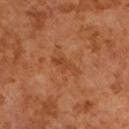notes = imaged on a skin check; not biopsied | illumination = cross-polarized | subject = male, approximately 65 years of age | size = ≈3.5 mm | acquisition = 15 mm crop, total-body photography.